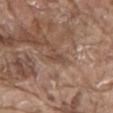notes — no biopsy performed (imaged during a skin exam); body site — the abdomen; acquisition — total-body-photography crop, ~15 mm field of view; diameter — ~2.5 mm (longest diameter); patient — male, roughly 80 years of age.